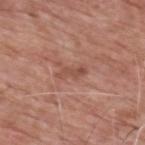Acquisition and patient details:
A male subject approximately 60 years of age. Cropped from a whole-body photographic skin survey; the tile spans about 15 mm. The recorded lesion diameter is about 3.5 mm. An algorithmic analysis of the crop reported a lesion color around L≈50 a*≈23 b*≈28 in CIELAB and a lesion–skin lightness drop of about 8. The software also gave a nevus-likeness score of about 0/100 and a detector confidence of about 95 out of 100 that the crop contains a lesion. Located on the upper back.